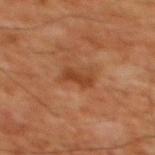The lesion-visualizer software estimated a footprint of about 3.5 mm² and an outline eccentricity of about 0.9 (0 = round, 1 = elongated). The analysis additionally found about 8 CIELAB-L* units darker than the surrounding skin. The analysis additionally found a border-irregularity index near 3/10 and a within-lesion color-variation index near 1/10.
The lesion is on the chest.
Approximately 3 mm at its widest.
Imaged with cross-polarized lighting.
A region of skin cropped from a whole-body photographic capture, roughly 15 mm wide.
A male patient aged approximately 60.